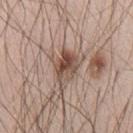Q: Was a biopsy performed?
A: imaged on a skin check; not biopsied
Q: What is the lesion's diameter?
A: about 4 mm
Q: What is the anatomic site?
A: the chest
Q: Automated lesion metrics?
A: border irregularity of about 3 on a 0–10 scale and a color-variation rating of about 9/10; an automated nevus-likeness rating near 90 out of 100 and lesion-presence confidence of about 100/100
Q: Who is the patient?
A: male, aged 43 to 47
Q: What kind of image is this?
A: 15 mm crop, total-body photography